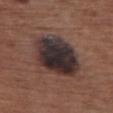Impression:
Recorded during total-body skin imaging; not selected for excision or biopsy.
Context:
Located on the chest. The subject is a female approximately 75 years of age. Cropped from a whole-body photographic skin survey; the tile spans about 15 mm.